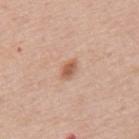Findings:
• notes: imaged on a skin check; not biopsied
• location: the mid back
• imaging modality: total-body-photography crop, ~15 mm field of view
• patient: male, aged 58 to 62
• tile lighting: white-light
• diameter: ~3 mm (longest diameter)